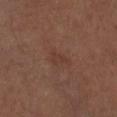Impression: Recorded during total-body skin imaging; not selected for excision or biopsy. Image and clinical context: From the leg. This is a white-light tile. A 15 mm crop from a total-body photograph taken for skin-cancer surveillance. A male patient aged 73 to 77.